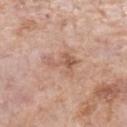Q: Was this lesion biopsied?
A: imaged on a skin check; not biopsied
Q: Lesion size?
A: ~4 mm (longest diameter)
Q: What is the imaging modality?
A: total-body-photography crop, ~15 mm field of view
Q: Who is the patient?
A: female, about 70 years old
Q: What is the anatomic site?
A: the left forearm